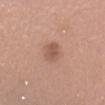Notes:
– workup: no biopsy performed (imaged during a skin exam)
– site: the head or neck
– imaging modality: 15 mm crop, total-body photography
– subject: female, roughly 35 years of age
– diameter: ~3 mm (longest diameter)